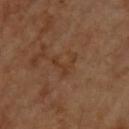Q: Where on the body is the lesion?
A: the upper back
Q: What is the lesion's diameter?
A: ~3 mm (longest diameter)
Q: What is the imaging modality?
A: ~15 mm tile from a whole-body skin photo